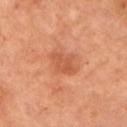body site: the left upper arm
lesion diameter: ~3.5 mm (longest diameter)
image source: ~15 mm tile from a whole-body skin photo
illumination: cross-polarized illumination
automated lesion analysis: a footprint of about 6.5 mm², an eccentricity of roughly 0.65, and a shape-asymmetry score of about 0.2 (0 = symmetric); a mean CIELAB color near L≈55 a*≈29 b*≈37, roughly 8 lightness units darker than nearby skin, and a normalized lesion–skin contrast near 5.5; lesion-presence confidence of about 100/100
subject: male, roughly 70 years of age